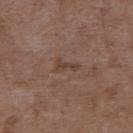Q: Was this lesion biopsied?
A: catalogued during a skin exam; not biopsied
Q: How was this image acquired?
A: 15 mm crop, total-body photography
Q: Illumination type?
A: white-light illumination
Q: What are the patient's age and sex?
A: male, aged approximately 50
Q: What is the anatomic site?
A: the right upper arm